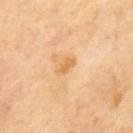Recorded during total-body skin imaging; not selected for excision or biopsy. The lesion is located on the mid back. A region of skin cropped from a whole-body photographic capture, roughly 15 mm wide.